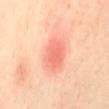The lesion was tiled from a total-body skin photograph and was not biopsied. A 15 mm crop from a total-body photograph taken for skin-cancer surveillance. The lesion's longest dimension is about 4.5 mm. The subject is a female aged approximately 40. The lesion is located on the mid back.